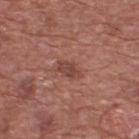Clinical impression:
Part of a total-body skin-imaging series; this lesion was reviewed on a skin check and was not flagged for biopsy.
Background:
The lesion is located on the upper back. A male patient, aged approximately 75. A 15 mm close-up extracted from a 3D total-body photography capture.